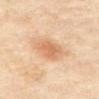workup = catalogued during a skin exam; not biopsied | anatomic site = the abdomen | size = ~4 mm (longest diameter) | illumination = cross-polarized illumination | patient = female, in their 50s | image source = ~15 mm crop, total-body skin-cancer survey | image-analysis metrics = a within-lesion color-variation index near 3/10 and radial color variation of about 1.